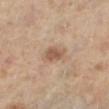Impression:
No biopsy was performed on this lesion — it was imaged during a full skin examination and was not determined to be concerning.
Acquisition and patient details:
A female subject, aged 58 to 62. A lesion tile, about 15 mm wide, cut from a 3D total-body photograph. The lesion-visualizer software estimated a lesion area of about 4.5 mm², a shape eccentricity near 0.7, and a shape-asymmetry score of about 0.25 (0 = symmetric). And it measured an average lesion color of about L≈54 a*≈18 b*≈29 (CIELAB) and a normalized border contrast of about 7. Captured under cross-polarized illumination. Located on the left lower leg.